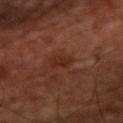No biopsy was performed on this lesion — it was imaged during a full skin examination and was not determined to be concerning.
This image is a 15 mm lesion crop taken from a total-body photograph.
The subject is in their mid- to late 60s.
The lesion is on the arm.
This is a cross-polarized tile.